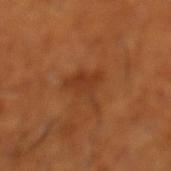Case summary:
• biopsy status · no biopsy performed (imaged during a skin exam)
• subject · male, about 65 years old
• tile lighting · cross-polarized illumination
• image · total-body-photography crop, ~15 mm field of view
• body site · the left lower leg